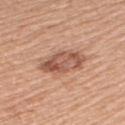Case summary:
– notes — imaged on a skin check; not biopsied
– size — ≈6 mm
– subject — female, about 65 years old
– imaging modality — ~15 mm tile from a whole-body skin photo
– site — the upper back
– lighting — white-light illumination
– TBP lesion metrics — a shape eccentricity near 0.9 and a symmetry-axis asymmetry near 0.2; internal color variation of about 6 on a 0–10 scale and radial color variation of about 2